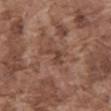Clinical impression: Recorded during total-body skin imaging; not selected for excision or biopsy. Background: Longest diameter approximately 3 mm. Captured under white-light illumination. The lesion is on the abdomen. Cropped from a whole-body photographic skin survey; the tile spans about 15 mm. A male patient approximately 75 years of age.